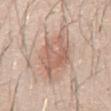Impression:
This lesion was catalogued during total-body skin photography and was not selected for biopsy.
Image and clinical context:
Cropped from a total-body skin-imaging series; the visible field is about 15 mm. An algorithmic analysis of the crop reported an area of roughly 23 mm², an outline eccentricity of about 0.85 (0 = round, 1 = elongated), and a shape-asymmetry score of about 0.3 (0 = symmetric). And it measured a mean CIELAB color near L≈62 a*≈18 b*≈27 and roughly 10 lightness units darker than nearby skin. And it measured a classifier nevus-likeness of about 10/100 and lesion-presence confidence of about 95/100. Located on the front of the torso. This is a white-light tile. Approximately 8 mm at its widest. A male patient about 30 years old.